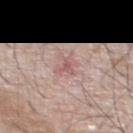Findings:
• notes · catalogued during a skin exam; not biopsied
• subject · male, in their 60s
• image · ~15 mm tile from a whole-body skin photo
• body site · the chest
• size · about 2.5 mm
• tile lighting · white-light illumination